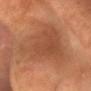follow-up: imaged on a skin check; not biopsied
size: ≈9 mm
subject: male, aged approximately 65
body site: the head or neck
image: 15 mm crop, total-body photography